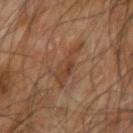notes: catalogued during a skin exam; not biopsied | lighting: cross-polarized illumination | body site: the left forearm | image source: ~15 mm crop, total-body skin-cancer survey | automated lesion analysis: a footprint of about 8 mm², an eccentricity of roughly 0.85, and a symmetry-axis asymmetry near 0.55; a lesion color around L≈43 a*≈20 b*≈31 in CIELAB, a lesion–skin lightness drop of about 7, and a lesion-to-skin contrast of about 6 (normalized; higher = more distinct); lesion-presence confidence of about 95/100 | patient: male, in their mid- to late 60s.